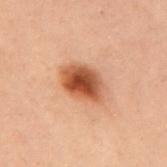notes = catalogued during a skin exam; not biopsied
diameter = about 5 mm
TBP lesion metrics = a lesion color around L≈45 a*≈23 b*≈32 in CIELAB, about 14 CIELAB-L* units darker than the surrounding skin, and a normalized border contrast of about 10.5; a classifier nevus-likeness of about 100/100
tile lighting = cross-polarized illumination
image source = ~15 mm tile from a whole-body skin photo
body site = the upper back
subject = female, in their mid- to late 50s